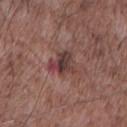biopsy status = no biopsy performed (imaged during a skin exam) | illumination = white-light | image source = total-body-photography crop, ~15 mm field of view | anatomic site = the front of the torso | diameter = about 3.5 mm | subject = male, aged approximately 70.